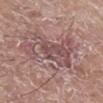Assessment: No biopsy was performed on this lesion — it was imaged during a full skin examination and was not determined to be concerning. Context: This is a white-light tile. The subject is a male aged 58–62. Measured at roughly 7.5 mm in maximum diameter. An algorithmic analysis of the crop reported a classifier nevus-likeness of about 0/100 and lesion-presence confidence of about 85/100. A roughly 15 mm field-of-view crop from a total-body skin photograph. The lesion is on the right lower leg.